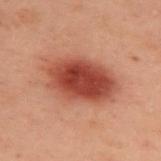Imaged during a routine full-body skin examination; the lesion was not biopsied and no histopathology is available. A female subject in their mid- to late 50s. On the upper back. Automated image analysis of the tile measured a footprint of about 26 mm², an eccentricity of roughly 0.8, and a shape-asymmetry score of about 0.15 (0 = symmetric). And it measured a classifier nevus-likeness of about 100/100 and a lesion-detection confidence of about 100/100. This image is a 15 mm lesion crop taken from a total-body photograph. Approximately 7.5 mm at its widest. The tile uses cross-polarized illumination.